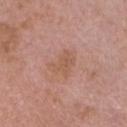This lesion was catalogued during total-body skin photography and was not selected for biopsy. A 15 mm crop from a total-body photograph taken for skin-cancer surveillance. A male subject aged approximately 75. This is a white-light tile. On the head or neck. About 3 mm across.